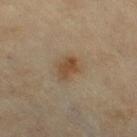Q: Is there a histopathology result?
A: no biopsy performed (imaged during a skin exam)
Q: What did automated image analysis measure?
A: border irregularity of about 2.5 on a 0–10 scale, internal color variation of about 3 on a 0–10 scale, and a peripheral color-asymmetry measure near 1; a classifier nevus-likeness of about 85/100 and lesion-presence confidence of about 100/100
Q: Who is the patient?
A: female, aged approximately 55
Q: Lesion location?
A: the right thigh
Q: How large is the lesion?
A: ~3 mm (longest diameter)
Q: Illumination type?
A: cross-polarized illumination
Q: How was this image acquired?
A: ~15 mm tile from a whole-body skin photo